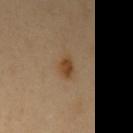The lesion was photographed on a routine skin check and not biopsied; there is no pathology result.
A male patient about 40 years old.
Captured under cross-polarized illumination.
The total-body-photography lesion software estimated a border-irregularity index near 2/10 and a within-lesion color-variation index near 3.5/10. It also reported an automated nevus-likeness rating near 95 out of 100.
A close-up tile cropped from a whole-body skin photograph, about 15 mm across.
From the left upper arm.
About 2.5 mm across.